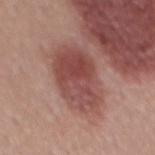Clinical impression: No biopsy was performed on this lesion — it was imaged during a full skin examination and was not determined to be concerning. Context: This is a white-light tile. A 15 mm close-up extracted from a 3D total-body photography capture. A male patient in their mid-50s. From the mid back.